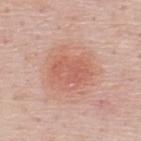Assessment:
Part of a total-body skin-imaging series; this lesion was reviewed on a skin check and was not flagged for biopsy.
Image and clinical context:
Automated tile analysis of the lesion measured an eccentricity of roughly 0.75 and a shape-asymmetry score of about 0.2 (0 = symmetric). And it measured a nevus-likeness score of about 80/100. A male patient aged approximately 50. Imaged with white-light lighting. Located on the upper back. A close-up tile cropped from a whole-body skin photograph, about 15 mm across. The lesion's longest dimension is about 4 mm.